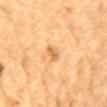Impression:
The lesion was tiled from a total-body skin photograph and was not biopsied.
Image and clinical context:
A male subject, aged 83 or older. A 15 mm crop from a total-body photograph taken for skin-cancer surveillance. About 2.5 mm across. The lesion is on the mid back.